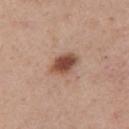The lesion was tiled from a total-body skin photograph and was not biopsied. On the chest. A male subject aged 73–77. A roughly 15 mm field-of-view crop from a total-body skin photograph.